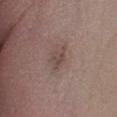biopsy_status: not biopsied; imaged during a skin examination
patient:
  sex: male
  age_approx: 65
image:
  source: total-body photography crop
  field_of_view_mm: 15
site: left lower leg
lesion_size:
  long_diameter_mm_approx: 3.0